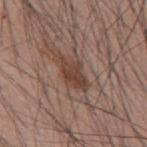workup: imaged on a skin check; not biopsied | image: 15 mm crop, total-body photography | illumination: white-light | automated metrics: an area of roughly 7.5 mm², a shape eccentricity near 0.85, and a shape-asymmetry score of about 0.25 (0 = symmetric); an average lesion color of about L≈42 a*≈19 b*≈26 (CIELAB) and about 10 CIELAB-L* units darker than the surrounding skin; a border-irregularity index near 3.5/10, internal color variation of about 2.5 on a 0–10 scale, and a peripheral color-asymmetry measure near 1 | location: the mid back | subject: male, roughly 60 years of age.